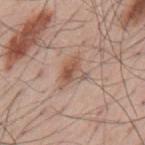Q: Was this lesion biopsied?
A: imaged on a skin check; not biopsied
Q: How was this image acquired?
A: total-body-photography crop, ~15 mm field of view
Q: Lesion location?
A: the mid back
Q: Patient demographics?
A: male, roughly 55 years of age